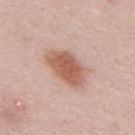Clinical impression: Captured during whole-body skin photography for melanoma surveillance; the lesion was not biopsied. Acquisition and patient details: The lesion is on the upper back. A male subject aged 23–27. A lesion tile, about 15 mm wide, cut from a 3D total-body photograph.